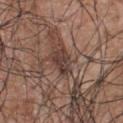Impression:
The lesion was tiled from a total-body skin photograph and was not biopsied.
Context:
The tile uses white-light illumination. About 3 mm across. Located on the upper back. A male subject, about 70 years old. Automated tile analysis of the lesion measured a footprint of about 5 mm² and an outline eccentricity of about 0.8 (0 = round, 1 = elongated). The analysis additionally found a lesion color around L≈38 a*≈17 b*≈21 in CIELAB, about 10 CIELAB-L* units darker than the surrounding skin, and a normalized border contrast of about 9. The software also gave a border-irregularity rating of about 3.5/10, internal color variation of about 2.5 on a 0–10 scale, and a peripheral color-asymmetry measure near 1. And it measured lesion-presence confidence of about 65/100. A 15 mm close-up tile from a total-body photography series done for melanoma screening.